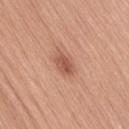Imaged during a routine full-body skin examination; the lesion was not biopsied and no histopathology is available. A female subject, in their mid-50s. The recorded lesion diameter is about 3 mm. Located on the lower back. This is a white-light tile. A roughly 15 mm field-of-view crop from a total-body skin photograph.